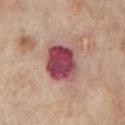<lesion>
<biopsy_status>not biopsied; imaged during a skin examination</biopsy_status>
<patient>
  <sex>male</sex>
  <age_approx>65</age_approx>
</patient>
<site>chest</site>
<lighting>white-light</lighting>
<automated_metrics>
  <cielab_L>47</cielab_L>
  <cielab_a>31</cielab_a>
  <cielab_b>18</cielab_b>
  <vs_skin_darker_L>18.0</vs_skin_darker_L>
  <vs_skin_contrast_norm>13.0</vs_skin_contrast_norm>
  <border_irregularity_0_10>1.5</border_irregularity_0_10>
  <peripheral_color_asymmetry>2.0</peripheral_color_asymmetry>
</automated_metrics>
<lesion_size>
  <long_diameter_mm_approx>4.5</long_diameter_mm_approx>
</lesion_size>
<image>
  <source>total-body photography crop</source>
  <field_of_view_mm>15</field_of_view_mm>
</image>
</lesion>